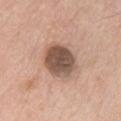biopsy_status: not biopsied; imaged during a skin examination
lighting: white-light
image:
  source: total-body photography crop
  field_of_view_mm: 15
patient:
  sex: male
  age_approx: 65
automated_metrics:
  area_mm2_approx: 15.0
  eccentricity: 0.55
  cielab_L: 52
  cielab_a: 17
  cielab_b: 26
  vs_skin_contrast_norm: 10.5
  border_irregularity_0_10: 1.0
  color_variation_0_10: 4.5
  peripheral_color_asymmetry: 1.5
site: chest